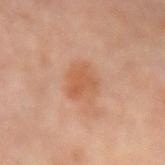Notes:
– notes: imaged on a skin check; not biopsied
– lighting: cross-polarized illumination
– diameter: ~4 mm (longest diameter)
– imaging modality: ~15 mm crop, total-body skin-cancer survey
– patient: male, aged approximately 65
– body site: the left forearm